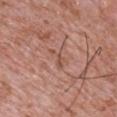Imaged during a routine full-body skin examination; the lesion was not biopsied and no histopathology is available. The recorded lesion diameter is about 3 mm. This is a white-light tile. The subject is a male roughly 45 years of age. Located on the chest. A region of skin cropped from a whole-body photographic capture, roughly 15 mm wide.